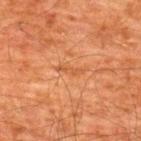Q: Was a biopsy performed?
A: catalogued during a skin exam; not biopsied